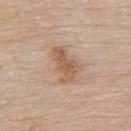Case summary:
- follow-up · catalogued during a skin exam; not biopsied
- tile lighting · white-light illumination
- body site · the upper back
- lesion diameter · about 4.5 mm
- automated metrics · an average lesion color of about L≈58 a*≈18 b*≈32 (CIELAB) and roughly 10 lightness units darker than nearby skin; a border-irregularity rating of about 4/10 and a peripheral color-asymmetry measure near 1; a classifier nevus-likeness of about 55/100 and a lesion-detection confidence of about 100/100
- acquisition · ~15 mm crop, total-body skin-cancer survey
- patient · male, approximately 85 years of age or older